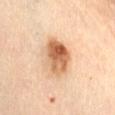Q: Was this lesion biopsied?
A: catalogued during a skin exam; not biopsied
Q: Who is the patient?
A: female, roughly 60 years of age
Q: How was this image acquired?
A: 15 mm crop, total-body photography
Q: What is the anatomic site?
A: the abdomen
Q: Lesion size?
A: ≈5 mm
Q: What did automated image analysis measure?
A: a shape eccentricity near 0.75; a mean CIELAB color near L≈64 a*≈22 b*≈38, a lesion–skin lightness drop of about 16, and a lesion-to-skin contrast of about 10 (normalized; higher = more distinct); a classifier nevus-likeness of about 100/100 and a lesion-detection confidence of about 100/100